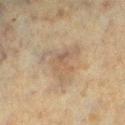Clinical impression:
The lesion was photographed on a routine skin check and not biopsied; there is no pathology result.
Acquisition and patient details:
A female subject about 55 years old. A 15 mm close-up extracted from a 3D total-body photography capture. Approximately 5.5 mm at its widest. An algorithmic analysis of the crop reported a nevus-likeness score of about 10/100 and a lesion-detection confidence of about 65/100. The lesion is located on the left lower leg.